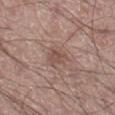<lesion>
  <biopsy_status>not biopsied; imaged during a skin examination</biopsy_status>
  <patient>
    <sex>male</sex>
    <age_approx>60</age_approx>
  </patient>
  <image>
    <source>total-body photography crop</source>
    <field_of_view_mm>15</field_of_view_mm>
  </image>
  <site>right lower leg</site>
</lesion>